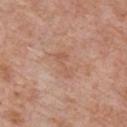Captured during whole-body skin photography for melanoma surveillance; the lesion was not biopsied. Measured at roughly 3.5 mm in maximum diameter. This is a white-light tile. This image is a 15 mm lesion crop taken from a total-body photograph. A male patient aged 58 to 62. The lesion is on the front of the torso.